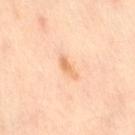workup = total-body-photography surveillance lesion; no biopsy | patient = female, approximately 45 years of age | imaging modality = ~15 mm tile from a whole-body skin photo | body site = the leg.